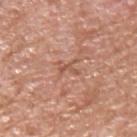<lesion>
  <biopsy_status>not biopsied; imaged during a skin examination</biopsy_status>
  <image>
    <source>total-body photography crop</source>
    <field_of_view_mm>15</field_of_view_mm>
  </image>
  <lesion_size>
    <long_diameter_mm_approx>2.5</long_diameter_mm_approx>
  </lesion_size>
  <automated_metrics>
    <vs_skin_darker_L>8.0</vs_skin_darker_L>
    <vs_skin_contrast_norm>5.5</vs_skin_contrast_norm>
    <nevus_likeness_0_100>0</nevus_likeness_0_100>
  </automated_metrics>
  <site>arm</site>
  <lighting>white-light</lighting>
  <patient>
    <sex>male</sex>
    <age_approx>60</age_approx>
  </patient>
</lesion>